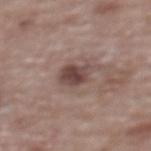Recorded during total-body skin imaging; not selected for excision or biopsy. A female subject in their mid- to late 60s. An algorithmic analysis of the crop reported a border-irregularity index near 3/10, a within-lesion color-variation index near 4.5/10, and peripheral color asymmetry of about 1.5. And it measured an automated nevus-likeness rating near 75 out of 100 and a detector confidence of about 100 out of 100 that the crop contains a lesion. This image is a 15 mm lesion crop taken from a total-body photograph. The lesion is on the upper back.